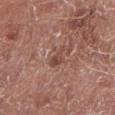Notes:
- biopsy status — no biopsy performed (imaged during a skin exam)
- lesion size — ~3 mm (longest diameter)
- location — the left lower leg
- lighting — white-light
- image source — ~15 mm tile from a whole-body skin photo
- patient — male, aged 63–67